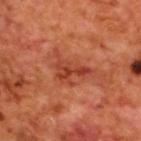Impression:
Captured during whole-body skin photography for melanoma surveillance; the lesion was not biopsied.
Background:
Located on the upper back. A 15 mm close-up tile from a total-body photography series done for melanoma screening. The recorded lesion diameter is about 5 mm. The patient is a male aged 68–72. Captured under cross-polarized illumination.